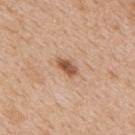{
  "biopsy_status": "not biopsied; imaged during a skin examination",
  "lighting": "white-light",
  "site": "back",
  "image": {
    "source": "total-body photography crop",
    "field_of_view_mm": 15
  },
  "lesion_size": {
    "long_diameter_mm_approx": 3.0
  },
  "patient": {
    "sex": "male",
    "age_approx": 65
  }
}